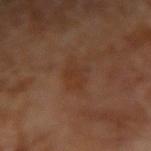The lesion's longest dimension is about 3.5 mm.
The total-body-photography lesion software estimated a nevus-likeness score of about 10/100 and a lesion-detection confidence of about 100/100.
Cropped from a whole-body photographic skin survey; the tile spans about 15 mm.
A male patient in their mid- to late 60s.
Imaged with cross-polarized lighting.